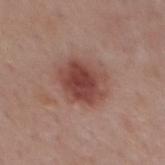Q: Is there a histopathology result?
A: total-body-photography surveillance lesion; no biopsy
Q: What is the anatomic site?
A: the mid back
Q: What kind of image is this?
A: ~15 mm crop, total-body skin-cancer survey
Q: What did automated image analysis measure?
A: an eccentricity of roughly 0.5 and a shape-asymmetry score of about 0.15 (0 = symmetric); roughly 12 lightness units darker than nearby skin and a normalized lesion–skin contrast near 9; border irregularity of about 1.5 on a 0–10 scale and a color-variation rating of about 4.5/10; a classifier nevus-likeness of about 95/100 and a detector confidence of about 100 out of 100 that the crop contains a lesion
Q: Patient demographics?
A: male, aged 53–57
Q: What is the lesion's diameter?
A: ≈5 mm